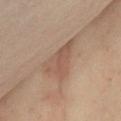Recorded during total-body skin imaging; not selected for excision or biopsy. About 6 mm across. From the chest. Cropped from a total-body skin-imaging series; the visible field is about 15 mm. A female subject, aged approximately 55. The tile uses cross-polarized illumination.